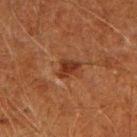Assessment:
This lesion was catalogued during total-body skin photography and was not selected for biopsy.
Image and clinical context:
A 15 mm crop from a total-body photograph taken for skin-cancer surveillance. The lesion is located on the right upper arm. A male patient aged approximately 60.